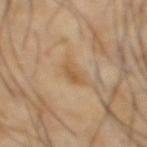This lesion was catalogued during total-body skin photography and was not selected for biopsy. This image is a 15 mm lesion crop taken from a total-body photograph. A male subject in their mid-60s. The lesion is on the abdomen.